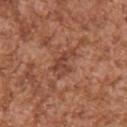Q: Is there a histopathology result?
A: total-body-photography surveillance lesion; no biopsy
Q: What kind of image is this?
A: ~15 mm crop, total-body skin-cancer survey
Q: What are the patient's age and sex?
A: male, approximately 45 years of age
Q: Lesion location?
A: the right upper arm